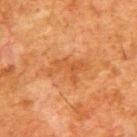Notes:
* notes — catalogued during a skin exam; not biopsied
* body site — the upper back
* image — ~15 mm crop, total-body skin-cancer survey
* patient — male, about 80 years old
* automated metrics — a lesion color around L≈44 a*≈23 b*≈36 in CIELAB and roughly 6 lightness units darker than nearby skin; a border-irregularity rating of about 5.5/10 and a peripheral color-asymmetry measure near 0.5; a detector confidence of about 100 out of 100 that the crop contains a lesion